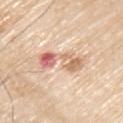| feature | finding |
|---|---|
| subject | male, aged around 80 |
| illumination | white-light |
| anatomic site | the left upper arm |
| lesion diameter | ~5.5 mm (longest diameter) |
| automated metrics | an outline eccentricity of about 0.95 (0 = round, 1 = elongated); an average lesion color of about L≈67 a*≈22 b*≈31 (CIELAB), roughly 13 lightness units darker than nearby skin, and a lesion-to-skin contrast of about 7.5 (normalized; higher = more distinct); border irregularity of about 6.5 on a 0–10 scale, a within-lesion color-variation index near 10/10, and a peripheral color-asymmetry measure near 6.5 |
| image source | ~15 mm tile from a whole-body skin photo |